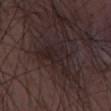workup: no biopsy performed (imaged during a skin exam) | image source: ~15 mm tile from a whole-body skin photo | patient: male, aged around 50 | tile lighting: white-light | size: about 5 mm | automated lesion analysis: a lesion area of about 13 mm² and a shape-asymmetry score of about 0.45 (0 = symmetric); a mean CIELAB color near L≈21 a*≈13 b*≈12; border irregularity of about 5.5 on a 0–10 scale and a within-lesion color-variation index near 3/10; a nevus-likeness score of about 0/100 and lesion-presence confidence of about 75/100.